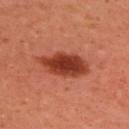Clinical impression:
Imaged during a routine full-body skin examination; the lesion was not biopsied and no histopathology is available.
Image and clinical context:
Located on the upper back. A female patient, roughly 40 years of age. The total-body-photography lesion software estimated a lesion color around L≈41 a*≈32 b*≈34 in CIELAB, roughly 15 lightness units darker than nearby skin, and a normalized border contrast of about 11. This is a cross-polarized tile. A 15 mm crop from a total-body photograph taken for skin-cancer surveillance. Measured at roughly 6.5 mm in maximum diameter.